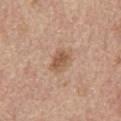Q: Was a biopsy performed?
A: catalogued during a skin exam; not biopsied